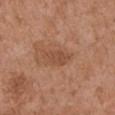The lesion was photographed on a routine skin check and not biopsied; there is no pathology result.
From the left upper arm.
A male subject aged around 75.
Imaged with white-light lighting.
About 3.5 mm across.
A 15 mm crop from a total-body photograph taken for skin-cancer surveillance.